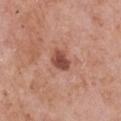Imaged during a routine full-body skin examination; the lesion was not biopsied and no histopathology is available. The patient is a female approximately 60 years of age. A 15 mm crop from a total-body photograph taken for skin-cancer surveillance. Imaged with white-light lighting. The lesion is on the front of the torso. The recorded lesion diameter is about 3 mm.